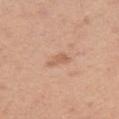follow-up: total-body-photography surveillance lesion; no biopsy | subject: male, aged around 45 | diameter: ~2.5 mm (longest diameter) | site: the left upper arm | imaging modality: ~15 mm tile from a whole-body skin photo | tile lighting: white-light.